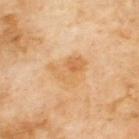Q: Automated lesion metrics?
A: a lesion–skin lightness drop of about 7 and a normalized lesion–skin contrast near 5.5; border irregularity of about 5 on a 0–10 scale and peripheral color asymmetry of about 2.5
Q: Lesion size?
A: ~4 mm (longest diameter)
Q: Lesion location?
A: the upper back
Q: Who is the patient?
A: male, aged 68 to 72
Q: What is the imaging modality?
A: ~15 mm crop, total-body skin-cancer survey